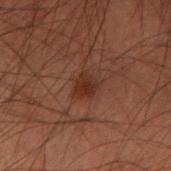<tbp_lesion>
<biopsy_status>not biopsied; imaged during a skin examination</biopsy_status>
</tbp_lesion>